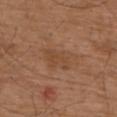biopsy status: no biopsy performed (imaged during a skin exam) | lighting: white-light illumination | TBP lesion metrics: an area of roughly 9 mm², an outline eccentricity of about 0.8 (0 = round, 1 = elongated), and two-axis asymmetry of about 0.35; a mean CIELAB color near L≈46 a*≈20 b*≈32, a lesion–skin lightness drop of about 6, and a normalized lesion–skin contrast near 5; a border-irregularity index near 4/10, a color-variation rating of about 2.5/10, and a peripheral color-asymmetry measure near 1 | lesion size: about 4.5 mm | body site: the back | patient: male, aged 73 to 77 | image: total-body-photography crop, ~15 mm field of view.